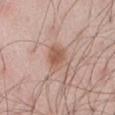– biopsy status — catalogued during a skin exam; not biopsied
– image — total-body-photography crop, ~15 mm field of view
– patient — male, aged 53–57
– illumination — white-light
– site — the abdomen